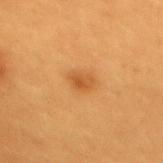• workup · total-body-photography surveillance lesion; no biopsy
• lighting · cross-polarized illumination
• patient · female, aged 28–32
• site · the mid back
• imaging modality · ~15 mm crop, total-body skin-cancer survey
• lesion size · about 2.5 mm